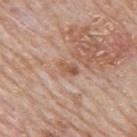This lesion was catalogued during total-body skin photography and was not selected for biopsy.
On the mid back.
A roughly 15 mm field-of-view crop from a total-body skin photograph.
A male patient about 80 years old.
The tile uses white-light illumination.
Measured at roughly 2.5 mm in maximum diameter.